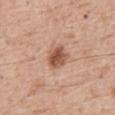Q: Was this lesion biopsied?
A: imaged on a skin check; not biopsied
Q: What is the anatomic site?
A: the abdomen
Q: Who is the patient?
A: male, aged 58 to 62
Q: What lighting was used for the tile?
A: white-light
Q: How was this image acquired?
A: ~15 mm tile from a whole-body skin photo
Q: Automated lesion metrics?
A: an area of roughly 7 mm² and a shape-asymmetry score of about 0.2 (0 = symmetric); internal color variation of about 4.5 on a 0–10 scale and peripheral color asymmetry of about 1.5
Q: How large is the lesion?
A: about 3.5 mm